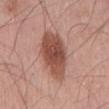The lesion was photographed on a routine skin check and not biopsied; there is no pathology result.
A 15 mm close-up extracted from a 3D total-body photography capture.
Measured at roughly 7 mm in maximum diameter.
A male patient aged 68 to 72.
Located on the mid back.